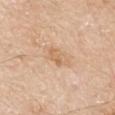{
  "biopsy_status": "not biopsied; imaged during a skin examination",
  "patient": {
    "sex": "male",
    "age_approx": 80
  },
  "image": {
    "source": "total-body photography crop",
    "field_of_view_mm": 15
  },
  "lighting": "white-light",
  "lesion_size": {
    "long_diameter_mm_approx": 3.0
  },
  "site": "left upper arm"
}